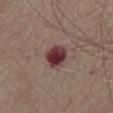follow-up: catalogued during a skin exam; not biopsied | patient: male, roughly 75 years of age | body site: the front of the torso | image: total-body-photography crop, ~15 mm field of view | tile lighting: white-light | size: about 3 mm.